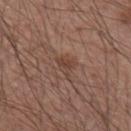<lesion>
<biopsy_status>not biopsied; imaged during a skin examination</biopsy_status>
<site>right upper arm</site>
<image>
  <source>total-body photography crop</source>
  <field_of_view_mm>15</field_of_view_mm>
</image>
<lighting>white-light</lighting>
<lesion_size>
  <long_diameter_mm_approx>3.0</long_diameter_mm_approx>
</lesion_size>
<automated_metrics>
  <border_irregularity_0_10>4.0</border_irregularity_0_10>
  <color_variation_0_10>2.5</color_variation_0_10>
  <peripheral_color_asymmetry>1.0</peripheral_color_asymmetry>
  <nevus_likeness_0_100>0</nevus_likeness_0_100>
  <lesion_detection_confidence_0_100>100</lesion_detection_confidence_0_100>
</automated_metrics>
<patient>
  <sex>male</sex>
  <age_approx>55</age_approx>
</patient>
</lesion>